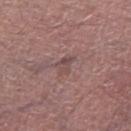The lesion was photographed on a routine skin check and not biopsied; there is no pathology result. From the left thigh. The recorded lesion diameter is about 2.5 mm. The tile uses white-light illumination. A lesion tile, about 15 mm wide, cut from a 3D total-body photograph. A male subject, approximately 70 years of age.